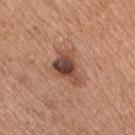Imaged during a routine full-body skin examination; the lesion was not biopsied and no histopathology is available.
Cropped from a whole-body photographic skin survey; the tile spans about 15 mm.
Captured under white-light illumination.
The total-body-photography lesion software estimated an average lesion color of about L≈47 a*≈22 b*≈27 (CIELAB), a lesion–skin lightness drop of about 14, and a normalized border contrast of about 10. The software also gave border irregularity of about 4.5 on a 0–10 scale, a color-variation rating of about 7.5/10, and peripheral color asymmetry of about 2.
A male subject, in their mid- to late 50s.
Approximately 5 mm at its widest.
From the front of the torso.